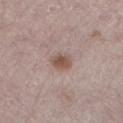Q: What kind of image is this?
A: ~15 mm crop, total-body skin-cancer survey
Q: How was the tile lit?
A: white-light illumination
Q: Automated lesion metrics?
A: a border-irregularity rating of about 2/10, internal color variation of about 2.5 on a 0–10 scale, and a peripheral color-asymmetry measure near 1
Q: Where on the body is the lesion?
A: the left lower leg
Q: Who is the patient?
A: male, in their mid-60s
Q: What is the lesion's diameter?
A: ~3 mm (longest diameter)